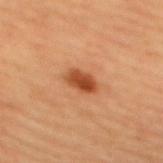Q: What is the anatomic site?
A: the upper back
Q: What is the imaging modality?
A: 15 mm crop, total-body photography
Q: How was the tile lit?
A: cross-polarized
Q: Who is the patient?
A: female, aged 43–47